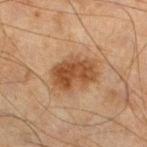No biopsy was performed on this lesion — it was imaged during a full skin examination and was not determined to be concerning. A region of skin cropped from a whole-body photographic capture, roughly 15 mm wide. The subject is a male in their mid- to late 40s. From the left lower leg.